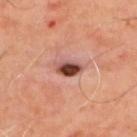anatomic site: the upper back | image-analysis metrics: border irregularity of about 2 on a 0–10 scale and a within-lesion color-variation index near 8.5/10 | diameter: ~3.5 mm (longest diameter) | subject: male, aged approximately 50 | acquisition: total-body-photography crop, ~15 mm field of view | lighting: cross-polarized.